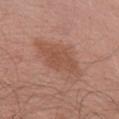workup: no biopsy performed (imaged during a skin exam)
illumination: white-light illumination
image: 15 mm crop, total-body photography
subject: male, in their mid-50s
size: ≈5 mm
body site: the right upper arm